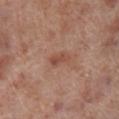Clinical impression: No biopsy was performed on this lesion — it was imaged during a full skin examination and was not determined to be concerning. Background: This is a white-light tile. From the leg. The patient is a male about 70 years old. A 15 mm crop from a total-body photograph taken for skin-cancer surveillance. Approximately 3 mm at its widest.